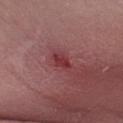The lesion was photographed on a routine skin check and not biopsied; there is no pathology result. Automated tile analysis of the lesion measured a lesion area of about 3 mm² and a shape-asymmetry score of about 0.2 (0 = symmetric). The software also gave an automated nevus-likeness rating near 5 out of 100 and a detector confidence of about 100 out of 100 that the crop contains a lesion. A lesion tile, about 15 mm wide, cut from a 3D total-body photograph. A male patient aged 48 to 52. The lesion is on the arm. The lesion's longest dimension is about 2.5 mm.